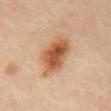The lesion was tiled from a total-body skin photograph and was not biopsied.
Longest diameter approximately 6 mm.
A 15 mm crop from a total-body photograph taken for skin-cancer surveillance.
From the abdomen.
The tile uses cross-polarized illumination.